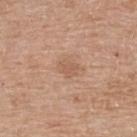Clinical impression:
Captured during whole-body skin photography for melanoma surveillance; the lesion was not biopsied.
Acquisition and patient details:
A female patient aged 58–62. Cropped from a total-body skin-imaging series; the visible field is about 15 mm. The recorded lesion diameter is about 2.5 mm. Automated image analysis of the tile measured an eccentricity of roughly 0.65. The analysis additionally found a border-irregularity rating of about 3/10 and a peripheral color-asymmetry measure near 0.5. It also reported a classifier nevus-likeness of about 0/100 and a lesion-detection confidence of about 100/100. The tile uses white-light illumination. Located on the upper back.